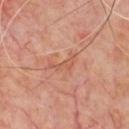follow-up: imaged on a skin check; not biopsied | imaging modality: 15 mm crop, total-body photography | anatomic site: the chest | subject: male, aged 63–67 | size: about 3.5 mm | illumination: cross-polarized.